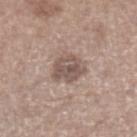biopsy_status: not biopsied; imaged during a skin examination
site: left lower leg
image:
  source: total-body photography crop
  field_of_view_mm: 15
lighting: white-light
patient:
  sex: male
  age_approx: 65
lesion_size:
  long_diameter_mm_approx: 4.0